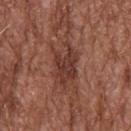notes: total-body-photography surveillance lesion; no biopsy | location: the upper back | patient: male, aged 73 to 77 | acquisition: ~15 mm tile from a whole-body skin photo.